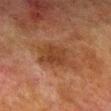Case summary:
- follow-up — catalogued during a skin exam; not biopsied
- tile lighting — cross-polarized
- image — ~15 mm tile from a whole-body skin photo
- automated lesion analysis — a footprint of about 13 mm² and a symmetry-axis asymmetry near 0.25; an average lesion color of about L≈32 a*≈19 b*≈28 (CIELAB), roughly 7 lightness units darker than nearby skin, and a normalized lesion–skin contrast near 7
- subject — male, aged around 80
- lesion size — ~4.5 mm (longest diameter)
- site — the right lower leg